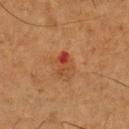Clinical impression:
No biopsy was performed on this lesion — it was imaged during a full skin examination and was not determined to be concerning.
Image and clinical context:
A 15 mm close-up extracted from a 3D total-body photography capture. A male patient aged 53 to 57. From the chest. The recorded lesion diameter is about 3 mm. The tile uses cross-polarized illumination.